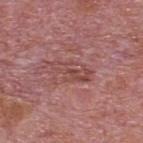Clinical summary: A male subject aged 73 to 77. A region of skin cropped from a whole-body photographic capture, roughly 15 mm wide. Located on the upper back. The tile uses white-light illumination. The lesion's longest dimension is about 5 mm.